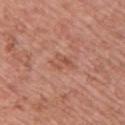Case summary:
- follow-up · total-body-photography surveillance lesion; no biopsy
- site · the upper back
- patient · female, approximately 40 years of age
- automated lesion analysis · a footprint of about 3.5 mm², an outline eccentricity of about 0.75 (0 = round, 1 = elongated), and a symmetry-axis asymmetry near 0.5; border irregularity of about 6 on a 0–10 scale and a within-lesion color-variation index near 0/10; a nevus-likeness score of about 0/100
- image · ~15 mm tile from a whole-body skin photo